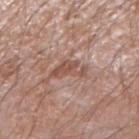Q: Was this lesion biopsied?
A: total-body-photography surveillance lesion; no biopsy
Q: How large is the lesion?
A: about 4 mm
Q: Patient demographics?
A: male, roughly 60 years of age
Q: What is the imaging modality?
A: 15 mm crop, total-body photography
Q: What is the anatomic site?
A: the right forearm
Q: How was the tile lit?
A: white-light